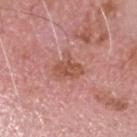Assessment: The lesion was photographed on a routine skin check and not biopsied; there is no pathology result. Background: A male patient aged around 75. The lesion is located on the head or neck. A 15 mm close-up tile from a total-body photography series done for melanoma screening.